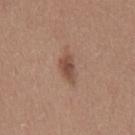Notes:
- workup — no biopsy performed (imaged during a skin exam)
- anatomic site — the chest
- subject — female, aged 23 to 27
- image-analysis metrics — a lesion area of about 4 mm² and two-axis asymmetry of about 0.3; a mean CIELAB color near L≈47 a*≈20 b*≈27, about 11 CIELAB-L* units darker than the surrounding skin, and a normalized border contrast of about 8; a border-irregularity rating of about 3/10, internal color variation of about 2.5 on a 0–10 scale, and peripheral color asymmetry of about 1; a classifier nevus-likeness of about 85/100 and a lesion-detection confidence of about 100/100
- diameter — ≈3 mm
- lighting — white-light illumination
- image — ~15 mm crop, total-body skin-cancer survey